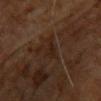Q: Is there a histopathology result?
A: imaged on a skin check; not biopsied
Q: What did automated image analysis measure?
A: a footprint of about 5 mm², an outline eccentricity of about 0.25 (0 = round, 1 = elongated), and two-axis asymmetry of about 0.45; about 5 CIELAB-L* units darker than the surrounding skin and a normalized border contrast of about 6.5; a classifier nevus-likeness of about 0/100 and a lesion-detection confidence of about 65/100
Q: Lesion size?
A: ≈2.5 mm
Q: Lesion location?
A: the upper back
Q: What are the patient's age and sex?
A: male, aged 58–62
Q: What is the imaging modality?
A: ~15 mm tile from a whole-body skin photo
Q: What lighting was used for the tile?
A: cross-polarized